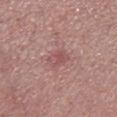notes = total-body-photography surveillance lesion; no biopsy | imaging modality = 15 mm crop, total-body photography | tile lighting = white-light | patient = female, in their 40s | anatomic site = the left lower leg | image-analysis metrics = an average lesion color of about L≈52 a*≈25 b*≈20 (CIELAB), roughly 7 lightness units darker than nearby skin, and a normalized border contrast of about 5.5; a classifier nevus-likeness of about 0/100 and lesion-presence confidence of about 100/100 | lesion diameter = ~2.5 mm (longest diameter).